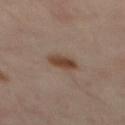notes = no biopsy performed (imaged during a skin exam) | patient = approximately 55 years of age | diameter = ~3 mm (longest diameter) | site = the mid back | image source = 15 mm crop, total-body photography | illumination = cross-polarized | automated lesion analysis = about 10 CIELAB-L* units darker than the surrounding skin and a normalized lesion–skin contrast near 9.5.